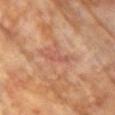Imaged during a routine full-body skin examination; the lesion was not biopsied and no histopathology is available.
The tile uses cross-polarized illumination.
A close-up tile cropped from a whole-body skin photograph, about 15 mm across.
The recorded lesion diameter is about 3 mm.
The subject is a female approximately 75 years of age.
On the back.
The lesion-visualizer software estimated a footprint of about 3.5 mm², an outline eccentricity of about 0.9 (0 = round, 1 = elongated), and a symmetry-axis asymmetry near 0.35. The software also gave roughly 7 lightness units darker than nearby skin and a lesion-to-skin contrast of about 5 (normalized; higher = more distinct). And it measured internal color variation of about 0.5 on a 0–10 scale and peripheral color asymmetry of about 0.